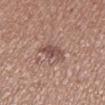From the left lower leg.
The patient is a female roughly 40 years of age.
Cropped from a whole-body photographic skin survey; the tile spans about 15 mm.
Automated image analysis of the tile measured a nevus-likeness score of about 10/100.
Longest diameter approximately 3.5 mm.
Imaged with white-light lighting.